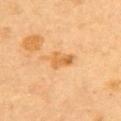patient: female, aged 58–62
site: the upper back
image: ~15 mm crop, total-body skin-cancer survey